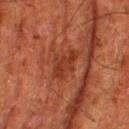The lesion was tiled from a total-body skin photograph and was not biopsied.
The lesion is on the right thigh.
The subject is a male aged around 80.
Approximately 3.5 mm at its widest.
A lesion tile, about 15 mm wide, cut from a 3D total-body photograph.
Imaged with cross-polarized lighting.